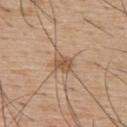<tbp_lesion>
<automated_metrics>
  <nevus_likeness_0_100>10</nevus_likeness_0_100>
  <lesion_detection_confidence_0_100>100</lesion_detection_confidence_0_100>
</automated_metrics>
<image>
  <source>total-body photography crop</source>
  <field_of_view_mm>15</field_of_view_mm>
</image>
<lighting>white-light</lighting>
<lesion_size>
  <long_diameter_mm_approx>3.0</long_diameter_mm_approx>
</lesion_size>
<patient>
  <sex>male</sex>
  <age_approx>55</age_approx>
</patient>
<site>upper back</site>
</tbp_lesion>